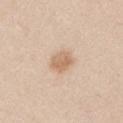Clinical impression: No biopsy was performed on this lesion — it was imaged during a full skin examination and was not determined to be concerning. Background: An algorithmic analysis of the crop reported a footprint of about 5.5 mm², an eccentricity of roughly 0.5, and a symmetry-axis asymmetry near 0.2. Captured under white-light illumination. The lesion is on the left upper arm. Approximately 3 mm at its widest. A 15 mm close-up extracted from a 3D total-body photography capture. A male patient, about 45 years old.